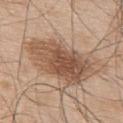This lesion was catalogued during total-body skin photography and was not selected for biopsy. A 15 mm crop from a total-body photograph taken for skin-cancer surveillance. The patient is a male in their mid-50s. The lesion is located on the upper back. Captured under white-light illumination. Approximately 8 mm at its widest.